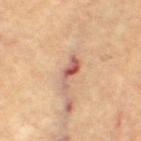{
  "biopsy_status": "not biopsied; imaged during a skin examination",
  "patient": {
    "sex": "female",
    "age_approx": 65
  },
  "automated_metrics": {
    "area_mm2_approx": 4.0
  },
  "image": {
    "source": "total-body photography crop",
    "field_of_view_mm": 15
  },
  "site": "left leg",
  "lesion_size": {
    "long_diameter_mm_approx": 4.0
  }
}